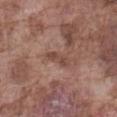patient = male, aged approximately 75; acquisition = ~15 mm tile from a whole-body skin photo; body site = the abdomen.